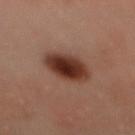Q: Is there a histopathology result?
A: total-body-photography surveillance lesion; no biopsy
Q: What kind of image is this?
A: ~15 mm crop, total-body skin-cancer survey
Q: What are the patient's age and sex?
A: female, approximately 50 years of age
Q: What lighting was used for the tile?
A: cross-polarized illumination
Q: What is the anatomic site?
A: the mid back
Q: What is the lesion's diameter?
A: about 5 mm